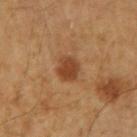<tbp_lesion>
  <biopsy_status>not biopsied; imaged during a skin examination</biopsy_status>
  <image>
    <source>total-body photography crop</source>
    <field_of_view_mm>15</field_of_view_mm>
  </image>
  <site>arm</site>
  <lesion_size>
    <long_diameter_mm_approx>3.0</long_diameter_mm_approx>
  </lesion_size>
  <patient>
    <sex>male</sex>
    <age_approx>60</age_approx>
  </patient>
</tbp_lesion>